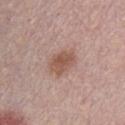Captured during whole-body skin photography for melanoma surveillance; the lesion was not biopsied.
This image is a 15 mm lesion crop taken from a total-body photograph.
A male subject roughly 30 years of age.
The lesion-visualizer software estimated a footprint of about 7.5 mm² and two-axis asymmetry of about 0.2. The software also gave an average lesion color of about L≈53 a*≈20 b*≈26 (CIELAB) and roughly 10 lightness units darker than nearby skin. And it measured a nevus-likeness score of about 55/100.
The tile uses white-light illumination.